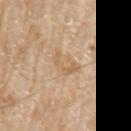follow-up=catalogued during a skin exam; not biopsied | anatomic site=the left upper arm | acquisition=~15 mm tile from a whole-body skin photo | patient=male, aged approximately 80.